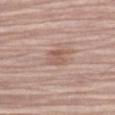Assessment: The lesion was photographed on a routine skin check and not biopsied; there is no pathology result. Context: A male subject, aged around 80. From the left upper arm. A 15 mm crop from a total-body photograph taken for skin-cancer surveillance. Captured under white-light illumination. Automated tile analysis of the lesion measured a mean CIELAB color near L≈57 a*≈19 b*≈26, about 8 CIELAB-L* units darker than the surrounding skin, and a normalized lesion–skin contrast near 5.5. And it measured border irregularity of about 2 on a 0–10 scale, internal color variation of about 3 on a 0–10 scale, and radial color variation of about 1. The analysis additionally found a classifier nevus-likeness of about 0/100 and a lesion-detection confidence of about 100/100. About 2.5 mm across.